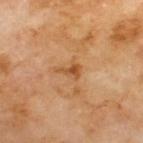{
  "biopsy_status": "not biopsied; imaged during a skin examination",
  "patient": {
    "sex": "male",
    "age_approx": 70
  },
  "lesion_size": {
    "long_diameter_mm_approx": 2.5
  },
  "site": "upper back",
  "image": {
    "source": "total-body photography crop",
    "field_of_view_mm": 15
  },
  "lighting": "cross-polarized"
}